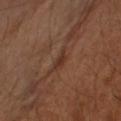Recorded during total-body skin imaging; not selected for excision or biopsy. A 15 mm close-up extracted from a 3D total-body photography capture. The lesion is located on the arm. Automated image analysis of the tile measured roughly 7 lightness units darker than nearby skin and a lesion-to-skin contrast of about 6.5 (normalized; higher = more distinct). The software also gave a classifier nevus-likeness of about 0/100 and a detector confidence of about 55 out of 100 that the crop contains a lesion. A male subject, about 50 years old.